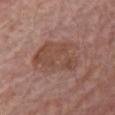<tbp_lesion>
  <biopsy_status>not biopsied; imaged during a skin examination</biopsy_status>
  <lighting>white-light</lighting>
  <lesion_size>
    <long_diameter_mm_approx>6.0</long_diameter_mm_approx>
  </lesion_size>
  <image>
    <source>total-body photography crop</source>
    <field_of_view_mm>15</field_of_view_mm>
  </image>
  <site>chest</site>
  <patient>
    <sex>male</sex>
    <age_approx>85+</age_approx>
  </patient>
</tbp_lesion>